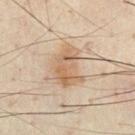A male subject aged 48 to 52. Located on the chest. A 15 mm crop from a total-body photograph taken for skin-cancer surveillance.